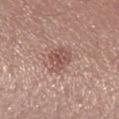{"biopsy_status": "not biopsied; imaged during a skin examination", "lighting": "white-light", "patient": {"sex": "female", "age_approx": 20}, "lesion_size": {"long_diameter_mm_approx": 3.5}, "image": {"source": "total-body photography crop", "field_of_view_mm": 15}, "site": "left lower leg", "automated_metrics": {"area_mm2_approx": 7.5, "eccentricity": 0.6}}